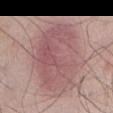This lesion was catalogued during total-body skin photography and was not selected for biopsy.
The subject is a male aged approximately 55.
Measured at roughly 8.5 mm in maximum diameter.
A 15 mm crop from a total-body photograph taken for skin-cancer surveillance.
Imaged with white-light lighting.
From the abdomen.